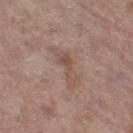biopsy status — no biopsy performed (imaged during a skin exam) | patient — female, aged approximately 85 or older | location — the leg | image-analysis metrics — a shape eccentricity near 0.95 and a symmetry-axis asymmetry near 0.4; an average lesion color of about L≈51 a*≈18 b*≈24 (CIELAB), a lesion–skin lightness drop of about 7, and a normalized lesion–skin contrast near 5.5; a border-irregularity index near 6.5/10, internal color variation of about 3 on a 0–10 scale, and peripheral color asymmetry of about 1; a classifier nevus-likeness of about 0/100 | lighting — white-light illumination | size — ~5 mm (longest diameter) | image source — ~15 mm tile from a whole-body skin photo.